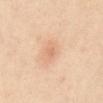- follow-up — no biopsy performed (imaged during a skin exam)
- acquisition — ~15 mm crop, total-body skin-cancer survey
- site — the abdomen
- patient — female, roughly 45 years of age